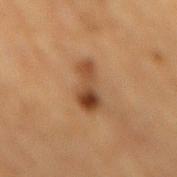Q: Was a biopsy performed?
A: imaged on a skin check; not biopsied
Q: What is the lesion's diameter?
A: ~5 mm (longest diameter)
Q: Automated lesion metrics?
A: an area of roughly 9 mm²; border irregularity of about 4 on a 0–10 scale, a color-variation rating of about 9/10, and radial color variation of about 3.5
Q: What kind of image is this?
A: total-body-photography crop, ~15 mm field of view
Q: How was the tile lit?
A: cross-polarized
Q: Patient demographics?
A: male, aged 83 or over
Q: What is the anatomic site?
A: the mid back